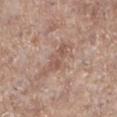Q: Is there a histopathology result?
A: imaged on a skin check; not biopsied